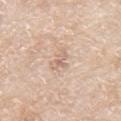Q: Who is the patient?
A: male, aged 78–82
Q: How was this image acquired?
A: ~15 mm tile from a whole-body skin photo
Q: How large is the lesion?
A: about 3 mm
Q: Illumination type?
A: white-light illumination
Q: Lesion location?
A: the mid back